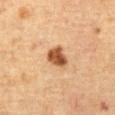Part of a total-body skin-imaging series; this lesion was reviewed on a skin check and was not flagged for biopsy. Located on the abdomen. A male patient in their mid-60s. This is a cross-polarized tile. A 15 mm crop from a total-body photograph taken for skin-cancer surveillance. The total-body-photography lesion software estimated border irregularity of about 2 on a 0–10 scale and a within-lesion color-variation index near 3.5/10.